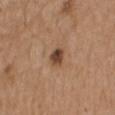The lesion was tiled from a total-body skin photograph and was not biopsied. The total-body-photography lesion software estimated a lesion area of about 4 mm², an outline eccentricity of about 0.65 (0 = round, 1 = elongated), and a shape-asymmetry score of about 0.25 (0 = symmetric). It also reported a mean CIELAB color near L≈45 a*≈19 b*≈30, about 13 CIELAB-L* units darker than the surrounding skin, and a normalized border contrast of about 10. The software also gave border irregularity of about 2.5 on a 0–10 scale, internal color variation of about 4.5 on a 0–10 scale, and a peripheral color-asymmetry measure near 1.5. It also reported a nevus-likeness score of about 95/100 and lesion-presence confidence of about 100/100. A male subject aged around 75. From the left upper arm. This is a white-light tile. This image is a 15 mm lesion crop taken from a total-body photograph. Measured at roughly 2.5 mm in maximum diameter.